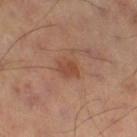Q: What lighting was used for the tile?
A: cross-polarized
Q: What is the imaging modality?
A: 15 mm crop, total-body photography
Q: Lesion size?
A: ≈2.5 mm
Q: Where on the body is the lesion?
A: the leg
Q: What did automated image analysis measure?
A: a mean CIELAB color near L≈48 a*≈24 b*≈31, a lesion–skin lightness drop of about 8, and a normalized lesion–skin contrast near 6; an automated nevus-likeness rating near 35 out of 100 and a lesion-detection confidence of about 100/100